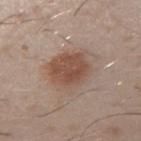Notes:
• notes · total-body-photography surveillance lesion; no biopsy
• image source · ~15 mm tile from a whole-body skin photo
• body site · the right upper arm
• patient · male, approximately 30 years of age
• lesion diameter · ~5 mm (longest diameter)
• tile lighting · white-light illumination
• image-analysis metrics · a border-irregularity index near 2/10, a within-lesion color-variation index near 3.5/10, and a peripheral color-asymmetry measure near 1